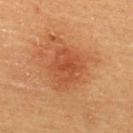{
  "biopsy_status": "not biopsied; imaged during a skin examination",
  "automated_metrics": {
    "area_mm2_approx": 11.0,
    "eccentricity": 0.55,
    "shape_asymmetry": 0.2
  },
  "site": "upper back",
  "image": {
    "source": "total-body photography crop",
    "field_of_view_mm": 15
  },
  "patient": {
    "sex": "male",
    "age_approx": 60
  },
  "lighting": "cross-polarized"
}